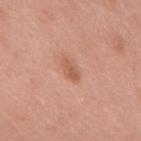Acquisition and patient details: The subject is a male about 50 years old. On the upper back. A 15 mm close-up tile from a total-body photography series done for melanoma screening. The lesion-visualizer software estimated a footprint of about 3.5 mm², an outline eccentricity of about 0.9 (0 = round, 1 = elongated), and a shape-asymmetry score of about 0.3 (0 = symmetric). And it measured a border-irregularity rating of about 3/10, a within-lesion color-variation index near 1/10, and radial color variation of about 0. The analysis additionally found a nevus-likeness score of about 25/100 and a detector confidence of about 100 out of 100 that the crop contains a lesion.